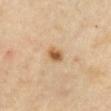Findings:
* biopsy status · imaged on a skin check; not biopsied
* illumination · cross-polarized
* image source · total-body-photography crop, ~15 mm field of view
* site · the arm
* lesion size · ≈2.5 mm
* patient · male, about 65 years old